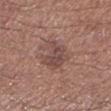This lesion was catalogued during total-body skin photography and was not selected for biopsy. From the left lower leg. A 15 mm crop from a total-body photograph taken for skin-cancer surveillance. The patient is a male aged 68–72.